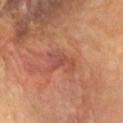The lesion is on the head or neck. A 15 mm crop from a total-body photograph taken for skin-cancer surveillance. The recorded lesion diameter is about 3.5 mm. The patient is a female roughly 65 years of age. This is a cross-polarized tile. Automated image analysis of the tile measured a shape eccentricity near 0.85 and two-axis asymmetry of about 0.45. And it measured an automated nevus-likeness rating near 0 out of 100 and a lesion-detection confidence of about 100/100.